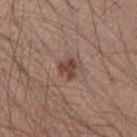Clinical impression: The lesion was photographed on a routine skin check and not biopsied; there is no pathology result. Acquisition and patient details: The subject is a male aged 38–42. The lesion is located on the right upper arm. Cropped from a whole-body photographic skin survey; the tile spans about 15 mm.